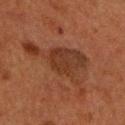workup=total-body-photography surveillance lesion; no biopsy
image=total-body-photography crop, ~15 mm field of view
subject=male, aged 58 to 62
site=the head or neck
tile lighting=cross-polarized
lesion diameter=about 8.5 mm